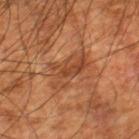biopsy status=imaged on a skin check; not biopsied | patient=male, aged 58–62 | image=~15 mm tile from a whole-body skin photo | body site=the right lower leg | size=~5 mm (longest diameter).